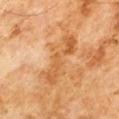follow-up = total-body-photography surveillance lesion; no biopsy
TBP lesion metrics = a footprint of about 15 mm², an eccentricity of roughly 0.9, and a symmetry-axis asymmetry near 0.4; a border-irregularity rating of about 6.5/10, a color-variation rating of about 5/10, and a peripheral color-asymmetry measure near 1.5; a nevus-likeness score of about 0/100 and lesion-presence confidence of about 100/100
patient = male, approximately 60 years of age
acquisition = ~15 mm tile from a whole-body skin photo
size = about 7 mm
illumination = cross-polarized illumination
site = the chest